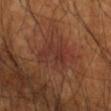This lesion was catalogued during total-body skin photography and was not selected for biopsy.
A male subject aged 63–67.
Longest diameter approximately 5 mm.
The lesion-visualizer software estimated a footprint of about 15 mm² and an eccentricity of roughly 0.65. It also reported an automated nevus-likeness rating near 0 out of 100 and lesion-presence confidence of about 100/100.
A lesion tile, about 15 mm wide, cut from a 3D total-body photograph.
Captured under cross-polarized illumination.
The lesion is on the left forearm.